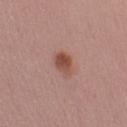Impression: This lesion was catalogued during total-body skin photography and was not selected for biopsy.